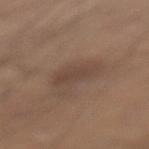  patient:
    sex: male
    age_approx: 30
  lesion_size:
    long_diameter_mm_approx: 4.5
  site: left lower leg
  automated_metrics:
    nevus_likeness_0_100: 30
    lesion_detection_confidence_0_100: 100
  image:
    source: total-body photography crop
    field_of_view_mm: 15
  lighting: white-light